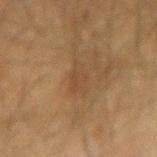Case summary:
– biopsy status · total-body-photography surveillance lesion; no biopsy
– illumination · cross-polarized
– lesion size · about 3.5 mm
– image · ~15 mm tile from a whole-body skin photo
– site · the arm
– subject · male, approximately 50 years of age
– automated lesion analysis · a footprint of about 4 mm² and an eccentricity of roughly 0.85; a mean CIELAB color near L≈36 a*≈14 b*≈27 and a lesion-to-skin contrast of about 4.5 (normalized; higher = more distinct); radial color variation of about 0